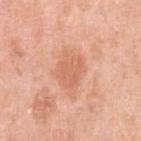image = ~15 mm tile from a whole-body skin photo | lesion diameter = ≈4 mm | subject = female, aged approximately 40 | location = the left upper arm.